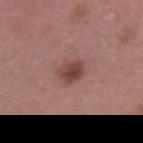| key | value |
|---|---|
| follow-up | imaged on a skin check; not biopsied |
| subject | female, aged 43–47 |
| acquisition | total-body-photography crop, ~15 mm field of view |
| site | the left upper arm |